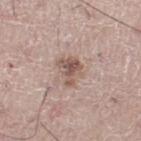Recorded during total-body skin imaging; not selected for excision or biopsy. A male patient about 70 years old. Imaged with white-light lighting. On the right leg. A roughly 15 mm field-of-view crop from a total-body skin photograph. Automated image analysis of the tile measured a border-irregularity rating of about 4/10, a color-variation rating of about 5/10, and a peripheral color-asymmetry measure near 1.5.